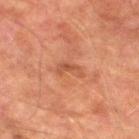notes: total-body-photography surveillance lesion; no biopsy
acquisition: ~15 mm crop, total-body skin-cancer survey
patient: male, aged around 75
site: the left lower leg
lesion diameter: ~3 mm (longest diameter)
lighting: cross-polarized